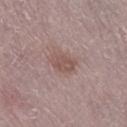Q: Was a biopsy performed?
A: catalogued during a skin exam; not biopsied
Q: What kind of image is this?
A: total-body-photography crop, ~15 mm field of view
Q: What is the lesion's diameter?
A: ≈3.5 mm
Q: What is the anatomic site?
A: the right lower leg
Q: Patient demographics?
A: female, aged 63 to 67
Q: Illumination type?
A: white-light illumination